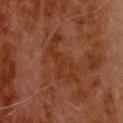Notes:
- workup · imaged on a skin check; not biopsied
- acquisition · 15 mm crop, total-body photography
- patient · male, aged 78 to 82
- body site · the upper back
- illumination · cross-polarized illumination